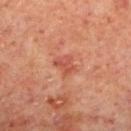Background: Located on the left lower leg. The tile uses cross-polarized illumination. A lesion tile, about 15 mm wide, cut from a 3D total-body photograph. A male patient, about 60 years old. Automated tile analysis of the lesion measured a classifier nevus-likeness of about 0/100.